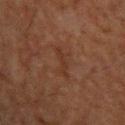Clinical impression:
No biopsy was performed on this lesion — it was imaged during a full skin examination and was not determined to be concerning.
Clinical summary:
The recorded lesion diameter is about 3.5 mm. Captured under cross-polarized illumination. The lesion-visualizer software estimated a lesion color around L≈25 a*≈17 b*≈23 in CIELAB and roughly 4 lightness units darker than nearby skin. A lesion tile, about 15 mm wide, cut from a 3D total-body photograph. The lesion is located on the upper back. A male patient, about 50 years old.